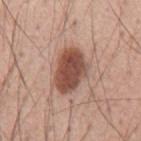follow-up = catalogued during a skin exam; not biopsied
body site = the mid back
imaging modality = total-body-photography crop, ~15 mm field of view
subject = male, aged approximately 55
tile lighting = white-light